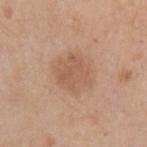Assessment:
No biopsy was performed on this lesion — it was imaged during a full skin examination and was not determined to be concerning.
Image and clinical context:
Cropped from a whole-body photographic skin survey; the tile spans about 15 mm. Automated image analysis of the tile measured a footprint of about 13 mm², an eccentricity of roughly 0.55, and a shape-asymmetry score of about 0.2 (0 = symmetric). It also reported an average lesion color of about L≈57 a*≈20 b*≈31 (CIELAB) and about 8 CIELAB-L* units darker than the surrounding skin. And it measured a border-irregularity rating of about 2.5/10, a color-variation rating of about 2.5/10, and a peripheral color-asymmetry measure near 1. And it measured a classifier nevus-likeness of about 10/100 and lesion-presence confidence of about 100/100. The subject is a male aged 58–62. The lesion is located on the left upper arm. This is a white-light tile.